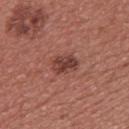Q: Was a biopsy performed?
A: imaged on a skin check; not biopsied
Q: Lesion location?
A: the upper back
Q: How was the tile lit?
A: white-light
Q: Who is the patient?
A: male, in their 40s
Q: How large is the lesion?
A: ~3 mm (longest diameter)
Q: What kind of image is this?
A: ~15 mm tile from a whole-body skin photo